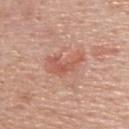biopsy_status: not biopsied; imaged during a skin examination
patient:
  sex: male
  age_approx: 60
image:
  source: total-body photography crop
  field_of_view_mm: 15
site: upper back